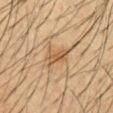{"biopsy_status": "not biopsied; imaged during a skin examination", "lesion_size": {"long_diameter_mm_approx": 3.5}, "lighting": "cross-polarized", "site": "abdomen", "automated_metrics": {"nevus_likeness_0_100": 25, "lesion_detection_confidence_0_100": 95}, "patient": {"sex": "male", "age_approx": 35}, "image": {"source": "total-body photography crop", "field_of_view_mm": 15}}